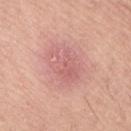The lesion was photographed on a routine skin check and not biopsied; there is no pathology result.
The lesion is located on the lower back.
Captured under white-light illumination.
A male patient roughly 65 years of age.
A 15 mm crop from a total-body photograph taken for skin-cancer surveillance.